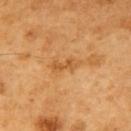Clinical impression:
Captured during whole-body skin photography for melanoma surveillance; the lesion was not biopsied.